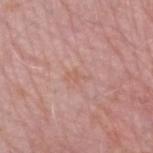No biopsy was performed on this lesion — it was imaged during a full skin examination and was not determined to be concerning.
The lesion-visualizer software estimated a footprint of about 2 mm² and an outline eccentricity of about 0.9 (0 = round, 1 = elongated). The software also gave a border-irregularity rating of about 5.5/10 and internal color variation of about 0 on a 0–10 scale. It also reported a nevus-likeness score of about 0/100 and lesion-presence confidence of about 100/100.
The patient is a male in their mid- to late 70s.
A 15 mm close-up extracted from a 3D total-body photography capture.
Imaged with white-light lighting.
About 2.5 mm across.
The lesion is located on the right forearm.